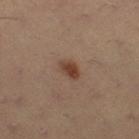Q: Was a biopsy performed?
A: total-body-photography surveillance lesion; no biopsy
Q: How was the tile lit?
A: cross-polarized
Q: How was this image acquired?
A: 15 mm crop, total-body photography
Q: Where on the body is the lesion?
A: the right thigh
Q: What are the patient's age and sex?
A: female, in their mid- to late 30s